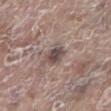Clinical impression:
This lesion was catalogued during total-body skin photography and was not selected for biopsy.
Acquisition and patient details:
Located on the left lower leg. A roughly 15 mm field-of-view crop from a total-body skin photograph. The total-body-photography lesion software estimated a nevus-likeness score of about 0/100 and a detector confidence of about 100 out of 100 that the crop contains a lesion. Measured at roughly 2.5 mm in maximum diameter. A female subject, aged 83 or older. This is a white-light tile.